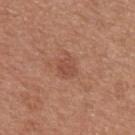| key | value |
|---|---|
| biopsy status | catalogued during a skin exam; not biopsied |
| patient | female, in their 50s |
| imaging modality | total-body-photography crop, ~15 mm field of view |
| site | the left upper arm |
| TBP lesion metrics | a mean CIELAB color near L≈49 a*≈24 b*≈30, a lesion–skin lightness drop of about 7, and a normalized border contrast of about 5.5; a border-irregularity index near 2/10, internal color variation of about 2 on a 0–10 scale, and peripheral color asymmetry of about 1 |